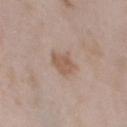Assessment:
The lesion was photographed on a routine skin check and not biopsied; there is no pathology result.
Context:
A 15 mm close-up extracted from a 3D total-body photography capture. Imaged with white-light lighting. On the chest. A female patient, roughly 30 years of age. About 3.5 mm across.